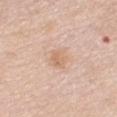Assessment: The lesion was photographed on a routine skin check and not biopsied; there is no pathology result. Image and clinical context: The patient is a female aged approximately 55. This is a white-light tile. A 15 mm crop from a total-body photograph taken for skin-cancer surveillance. Measured at roughly 2.5 mm in maximum diameter. The lesion is located on the chest.